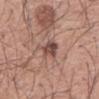notes: total-body-photography surveillance lesion; no biopsy
illumination: white-light illumination
patient: male, aged 53 to 57
body site: the mid back
image source: ~15 mm tile from a whole-body skin photo
TBP lesion metrics: a footprint of about 6 mm² and two-axis asymmetry of about 0.35; roughly 11 lightness units darker than nearby skin; a classifier nevus-likeness of about 30/100 and lesion-presence confidence of about 100/100
diameter: ≈4 mm